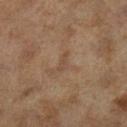Notes:
- subject: female, roughly 60 years of age
- acquisition: ~15 mm crop, total-body skin-cancer survey
- body site: the left lower leg
- lesion size: about 2.5 mm